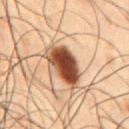biopsy_status: not biopsied; imaged during a skin examination
site: front of the torso
patient:
  sex: male
  age_approx: 50
lesion_size:
  long_diameter_mm_approx: 5.5
image:
  source: total-body photography crop
  field_of_view_mm: 15
lighting: cross-polarized